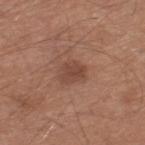Part of a total-body skin-imaging series; this lesion was reviewed on a skin check and was not flagged for biopsy.
Imaged with white-light lighting.
A roughly 15 mm field-of-view crop from a total-body skin photograph.
About 3 mm across.
A male patient, roughly 65 years of age.
The lesion is on the right lower leg.